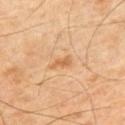Acquisition and patient details: The lesion-visualizer software estimated a footprint of about 3 mm² and an outline eccentricity of about 0.9 (0 = round, 1 = elongated). And it measured a color-variation rating of about 1/10 and radial color variation of about 0. It also reported a classifier nevus-likeness of about 0/100 and lesion-presence confidence of about 100/100. The patient is a male approximately 45 years of age. A close-up tile cropped from a whole-body skin photograph, about 15 mm across. This is a cross-polarized tile. From the left upper arm.